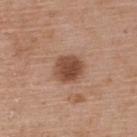This lesion was catalogued during total-body skin photography and was not selected for biopsy. The tile uses white-light illumination. Longest diameter approximately 3.5 mm. Located on the back. A close-up tile cropped from a whole-body skin photograph, about 15 mm across. The patient is a female approximately 60 years of age. The lesion-visualizer software estimated roughly 13 lightness units darker than nearby skin and a lesion-to-skin contrast of about 9.5 (normalized; higher = more distinct). And it measured lesion-presence confidence of about 100/100.